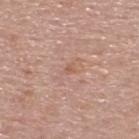Clinical summary: The tile uses white-light illumination. An algorithmic analysis of the crop reported internal color variation of about 0 on a 0–10 scale and a peripheral color-asymmetry measure near 0. And it measured a classifier nevus-likeness of about 0/100. On the back. About 1 mm across. A male patient, approximately 55 years of age. A close-up tile cropped from a whole-body skin photograph, about 15 mm across.